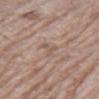Impression: The lesion was tiled from a total-body skin photograph and was not biopsied. Clinical summary: A close-up tile cropped from a whole-body skin photograph, about 15 mm across. The tile uses white-light illumination. A male subject approximately 65 years of age. On the right thigh.